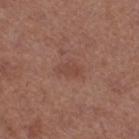Automated tile analysis of the lesion measured an outline eccentricity of about 0.7 (0 = round, 1 = elongated). The analysis additionally found a border-irregularity index near 3.5/10 and a within-lesion color-variation index near 2.5/10. The subject is a female about 50 years old. From the right thigh. The tile uses white-light illumination. Longest diameter approximately 3 mm. A 15 mm crop from a total-body photograph taken for skin-cancer surveillance.